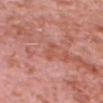No biopsy was performed on this lesion — it was imaged during a full skin examination and was not determined to be concerning. Automated tile analysis of the lesion measured a lesion color around L≈55 a*≈28 b*≈31 in CIELAB, about 6 CIELAB-L* units darker than the surrounding skin, and a normalized border contrast of about 5.5. A male subject, in their 80s. Captured under white-light illumination. Located on the head or neck. A 15 mm close-up tile from a total-body photography series done for melanoma screening. Approximately 2.5 mm at its widest.